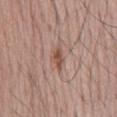Impression:
Captured during whole-body skin photography for melanoma surveillance; the lesion was not biopsied.
Clinical summary:
This is a white-light tile. A male patient, in their mid- to late 60s. A lesion tile, about 15 mm wide, cut from a 3D total-body photograph. The lesion is on the back.